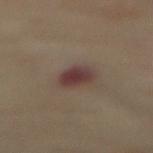Assessment: Part of a total-body skin-imaging series; this lesion was reviewed on a skin check and was not flagged for biopsy. Image and clinical context: Automated tile analysis of the lesion measured an area of roughly 6 mm² and an outline eccentricity of about 0.65 (0 = round, 1 = elongated). Measured at roughly 3.5 mm in maximum diameter. A female subject aged around 60. On the front of the torso. A roughly 15 mm field-of-view crop from a total-body skin photograph. The tile uses cross-polarized illumination.